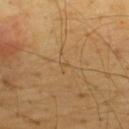{"image": {"source": "total-body photography crop", "field_of_view_mm": 15}, "site": "upper back", "lighting": "cross-polarized", "patient": {"sex": "male", "age_approx": 65}, "lesion_size": {"long_diameter_mm_approx": 1.0}}